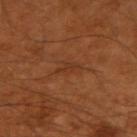<case>
  <biopsy_status>not biopsied; imaged during a skin examination</biopsy_status>
  <image>
    <source>total-body photography crop</source>
    <field_of_view_mm>15</field_of_view_mm>
  </image>
  <site>left arm</site>
  <lesion_size>
    <long_diameter_mm_approx>3.0</long_diameter_mm_approx>
  </lesion_size>
  <patient>
    <sex>male</sex>
    <age_approx>50</age_approx>
  </patient>
  <lighting>cross-polarized</lighting>
</case>